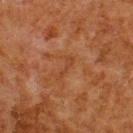Q: Was this lesion biopsied?
A: imaged on a skin check; not biopsied
Q: What is the anatomic site?
A: the back
Q: What are the patient's age and sex?
A: male, aged 78–82
Q: What did automated image analysis measure?
A: roughly 5 lightness units darker than nearby skin and a normalized border contrast of about 4.5; a within-lesion color-variation index near 0/10
Q: Illumination type?
A: cross-polarized
Q: How large is the lesion?
A: about 3 mm
Q: What kind of image is this?
A: 15 mm crop, total-body photography